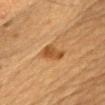lesion diameter = ≈3.5 mm; acquisition = ~15 mm tile from a whole-body skin photo; automated metrics = a lesion color around L≈45 a*≈21 b*≈38 in CIELAB and a normalized border contrast of about 8; patient = male, about 85 years old or older; anatomic site = the chest; lighting = cross-polarized illumination.